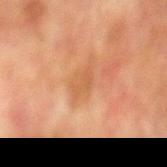{"biopsy_status": "not biopsied; imaged during a skin examination", "site": "mid back", "patient": {"sex": "male", "age_approx": 75}, "image": {"source": "total-body photography crop", "field_of_view_mm": 15}}